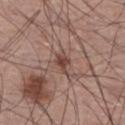This lesion was catalogued during total-body skin photography and was not selected for biopsy. A region of skin cropped from a whole-body photographic capture, roughly 15 mm wide. A male subject aged 58 to 62. From the right lower leg. Automated image analysis of the tile measured a border-irregularity index near 4.5/10. The analysis additionally found an automated nevus-likeness rating near 35 out of 100 and lesion-presence confidence of about 100/100.